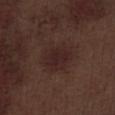<lesion>
  <biopsy_status>not biopsied; imaged during a skin examination</biopsy_status>
  <site>leg</site>
  <image>
    <source>total-body photography crop</source>
    <field_of_view_mm>15</field_of_view_mm>
  </image>
  <patient>
    <sex>male</sex>
    <age_approx>70</age_approx>
  </patient>
</lesion>